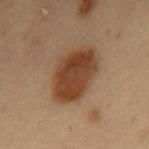biopsy status: total-body-photography surveillance lesion; no biopsy
body site: the mid back
TBP lesion metrics: a lesion area of about 19 mm², an outline eccentricity of about 0.8 (0 = round, 1 = elongated), and a symmetry-axis asymmetry near 0.15; a mean CIELAB color near L≈33 a*≈17 b*≈27 and a lesion-to-skin contrast of about 11 (normalized; higher = more distinct)
lesion size: ~6.5 mm (longest diameter)
patient: male, aged 53 to 57
image: ~15 mm tile from a whole-body skin photo